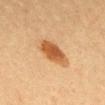Part of a total-body skin-imaging series; this lesion was reviewed on a skin check and was not flagged for biopsy. On the head or neck. The lesion-visualizer software estimated a footprint of about 9 mm², a shape eccentricity near 0.8, and two-axis asymmetry of about 0.2. It also reported a normalized border contrast of about 9.5. The software also gave a border-irregularity index near 2/10, a within-lesion color-variation index near 3.5/10, and radial color variation of about 1. The lesion's longest dimension is about 4 mm. The patient is a female aged 18 to 22. A close-up tile cropped from a whole-body skin photograph, about 15 mm across.